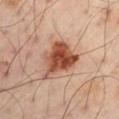Assessment:
The lesion was tiled from a total-body skin photograph and was not biopsied.
Context:
On the left arm. A close-up tile cropped from a whole-body skin photograph, about 15 mm across. A male subject, aged 48–52.